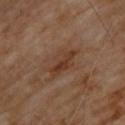follow-up: no biopsy performed (imaged during a skin exam)
image source: ~15 mm crop, total-body skin-cancer survey
illumination: cross-polarized
subject: male, aged 68–72
anatomic site: the upper back